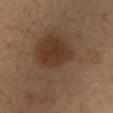Notes:
– biopsy status · catalogued during a skin exam; not biopsied
– image · ~15 mm tile from a whole-body skin photo
– subject · male, aged around 35
– illumination · cross-polarized
– body site · the left upper arm
– lesion diameter · ~18 mm (longest diameter)